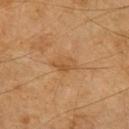{
  "biopsy_status": "not biopsied; imaged during a skin examination"
}